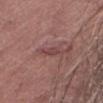Q: Was a biopsy performed?
A: no biopsy performed (imaged during a skin exam)
Q: What is the anatomic site?
A: the leg
Q: Automated lesion metrics?
A: an average lesion color of about L≈43 a*≈24 b*≈20 (CIELAB), about 8 CIELAB-L* units darker than the surrounding skin, and a normalized lesion–skin contrast near 6; border irregularity of about 4 on a 0–10 scale, a within-lesion color-variation index near 1/10, and peripheral color asymmetry of about 0; an automated nevus-likeness rating near 0 out of 100 and a lesion-detection confidence of about 60/100
Q: What is the lesion's diameter?
A: ~2.5 mm (longest diameter)
Q: Who is the patient?
A: male, approximately 75 years of age
Q: What is the imaging modality?
A: total-body-photography crop, ~15 mm field of view
Q: What lighting was used for the tile?
A: white-light illumination